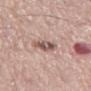Captured during whole-body skin photography for melanoma surveillance; the lesion was not biopsied. The lesion-visualizer software estimated a lesion area of about 5.5 mm², an eccentricity of roughly 0.65, and a shape-asymmetry score of about 0.3 (0 = symmetric). And it measured border irregularity of about 3 on a 0–10 scale, a within-lesion color-variation index near 6.5/10, and a peripheral color-asymmetry measure near 2.5. It also reported a nevus-likeness score of about 10/100. A male subject, aged approximately 75. Located on the left thigh. Measured at roughly 3 mm in maximum diameter. A 15 mm crop from a total-body photograph taken for skin-cancer surveillance.